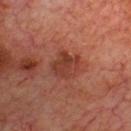Acquisition and patient details:
Imaged with cross-polarized lighting. A male patient roughly 70 years of age. The lesion-visualizer software estimated an area of roughly 9 mm², a shape eccentricity near 0.45, and two-axis asymmetry of about 0.2. It also reported a normalized border contrast of about 7. And it measured a border-irregularity rating of about 2.5/10, internal color variation of about 3 on a 0–10 scale, and radial color variation of about 1. And it measured a nevus-likeness score of about 20/100 and a detector confidence of about 100 out of 100 that the crop contains a lesion. Cropped from a whole-body photographic skin survey; the tile spans about 15 mm. On the chest.